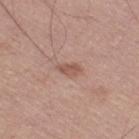biopsy status = imaged on a skin check; not biopsied | location = the right thigh | image = total-body-photography crop, ~15 mm field of view | automated lesion analysis = a lesion color around L≈54 a*≈21 b*≈26 in CIELAB and a normalized lesion–skin contrast near 6.5; border irregularity of about 2.5 on a 0–10 scale and a within-lesion color-variation index near 1.5/10 | size = ~2.5 mm (longest diameter) | subject = male, aged approximately 50 | illumination = white-light illumination.